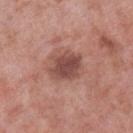Impression: This lesion was catalogued during total-body skin photography and was not selected for biopsy. Image and clinical context: This is a white-light tile. A lesion tile, about 15 mm wide, cut from a 3D total-body photograph. On the left lower leg. The subject is a male aged around 55.